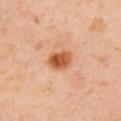Clinical impression: This lesion was catalogued during total-body skin photography and was not selected for biopsy. Image and clinical context: This is a cross-polarized tile. Automated image analysis of the tile measured a footprint of about 6 mm², a shape eccentricity near 0.5, and two-axis asymmetry of about 0.2. And it measured a mean CIELAB color near L≈56 a*≈29 b*≈40, roughly 15 lightness units darker than nearby skin, and a lesion-to-skin contrast of about 10.5 (normalized; higher = more distinct). A 15 mm close-up tile from a total-body photography series done for melanoma screening. A female patient approximately 40 years of age. The lesion is on the right upper arm. About 3 mm across.